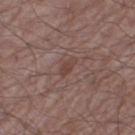Q: Was this lesion biopsied?
A: catalogued during a skin exam; not biopsied
Q: Lesion location?
A: the right thigh
Q: What is the lesion's diameter?
A: about 2.5 mm
Q: What is the imaging modality?
A: ~15 mm tile from a whole-body skin photo
Q: Illumination type?
A: white-light illumination
Q: Who is the patient?
A: male, aged 63 to 67